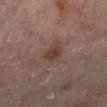Findings:
- workup — total-body-photography surveillance lesion; no biopsy
- location — the right lower leg
- image source — ~15 mm tile from a whole-body skin photo
- illumination — cross-polarized illumination
- diameter — ~3 mm (longest diameter)
- subject — male, about 65 years old
- automated metrics — a classifier nevus-likeness of about 45/100 and a detector confidence of about 100 out of 100 that the crop contains a lesion